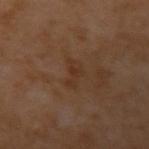Impression:
The lesion was tiled from a total-body skin photograph and was not biopsied.
Acquisition and patient details:
The lesion's longest dimension is about 3 mm. Captured under cross-polarized illumination. A close-up tile cropped from a whole-body skin photograph, about 15 mm across. On the left upper arm. The subject is a male aged 53 to 57.